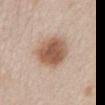{
  "biopsy_status": "not biopsied; imaged during a skin examination",
  "patient": {
    "sex": "male",
    "age_approx": 60
  },
  "lighting": "white-light",
  "lesion_size": {
    "long_diameter_mm_approx": 5.0
  },
  "automated_metrics": {
    "area_mm2_approx": 15.0,
    "shape_asymmetry": 0.2,
    "cielab_L": 56,
    "cielab_a": 19,
    "cielab_b": 29,
    "vs_skin_darker_L": 14.0,
    "vs_skin_contrast_norm": 10.0,
    "border_irregularity_0_10": 2.0,
    "peripheral_color_asymmetry": 1.5
  },
  "image": {
    "source": "total-body photography crop",
    "field_of_view_mm": 15
  },
  "site": "abdomen"
}